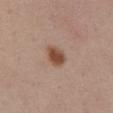This lesion was catalogued during total-body skin photography and was not selected for biopsy. Cropped from a whole-body photographic skin survey; the tile spans about 15 mm. A female patient aged approximately 55. The lesion is located on the abdomen. Automated tile analysis of the lesion measured a lesion area of about 5.5 mm². And it measured a nevus-likeness score of about 100/100 and a lesion-detection confidence of about 100/100.